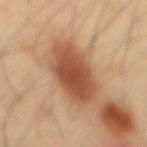Part of a total-body skin-imaging series; this lesion was reviewed on a skin check and was not flagged for biopsy.
An algorithmic analysis of the crop reported a footprint of about 23 mm², an eccentricity of roughly 0.75, and a symmetry-axis asymmetry near 0.1. The software also gave a classifier nevus-likeness of about 100/100 and lesion-presence confidence of about 100/100.
This image is a 15 mm lesion crop taken from a total-body photograph.
Imaged with cross-polarized lighting.
The recorded lesion diameter is about 6.5 mm.
The lesion is on the mid back.
The subject is a male approximately 40 years of age.